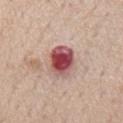Notes:
* follow-up — catalogued during a skin exam; not biopsied
* site — the mid back
* image — ~15 mm tile from a whole-body skin photo
* image-analysis metrics — a lesion area of about 10 mm², a shape eccentricity near 0.75, and a symmetry-axis asymmetry near 0.15; a lesion color around L≈50 a*≈30 b*≈21 in CIELAB, roughly 19 lightness units darker than nearby skin, and a lesion-to-skin contrast of about 13 (normalized; higher = more distinct); border irregularity of about 1.5 on a 0–10 scale
* patient — male, aged around 70